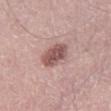Clinical impression:
Recorded during total-body skin imaging; not selected for excision or biopsy.
Context:
The lesion is located on the right thigh. A male subject, aged 58 to 62. Cropped from a total-body skin-imaging series; the visible field is about 15 mm.